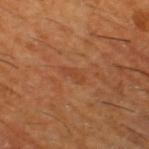Assessment: Captured during whole-body skin photography for melanoma surveillance; the lesion was not biopsied. Context: A close-up tile cropped from a whole-body skin photograph, about 15 mm across. The lesion is on the left thigh. The patient is a male aged approximately 60. Longest diameter approximately 2.5 mm. The total-body-photography lesion software estimated border irregularity of about 3.5 on a 0–10 scale, a color-variation rating of about 0/10, and peripheral color asymmetry of about 0. This is a cross-polarized tile.